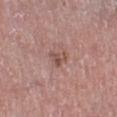Clinical impression:
Imaged during a routine full-body skin examination; the lesion was not biopsied and no histopathology is available.
Background:
Captured under white-light illumination. From the left lower leg. A male patient aged approximately 75. Approximately 2.5 mm at its widest. This image is a 15 mm lesion crop taken from a total-body photograph.